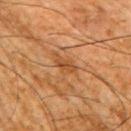{"biopsy_status": "not biopsied; imaged during a skin examination", "patient": {"sex": "male", "age_approx": 65}, "lighting": "cross-polarized", "automated_metrics": {"border_irregularity_0_10": 2.5, "color_variation_0_10": 2.0, "nevus_likeness_0_100": 0}, "image": {"source": "total-body photography crop", "field_of_view_mm": 15}, "site": "arm", "lesion_size": {"long_diameter_mm_approx": 2.5}}